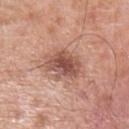notes — no biopsy performed (imaged during a skin exam) | size — ~4 mm (longest diameter) | image — 15 mm crop, total-body photography | subject — male, roughly 80 years of age | lighting — white-light illumination | body site — the left lower leg.